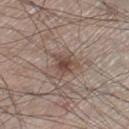About 3.5 mm across. The tile uses white-light illumination. From the right lower leg. A close-up tile cropped from a whole-body skin photograph, about 15 mm across. The patient is a male aged 58–62.